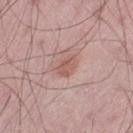Q: Is there a histopathology result?
A: total-body-photography surveillance lesion; no biopsy
Q: What lighting was used for the tile?
A: white-light
Q: How was this image acquired?
A: ~15 mm crop, total-body skin-cancer survey
Q: What did automated image analysis measure?
A: a lesion color around L≈57 a*≈22 b*≈25 in CIELAB and a lesion-to-skin contrast of about 6 (normalized; higher = more distinct)
Q: Who is the patient?
A: male, aged 43–47
Q: Where on the body is the lesion?
A: the right thigh
Q: Lesion size?
A: ~2.5 mm (longest diameter)